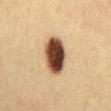Recorded during total-body skin imaging; not selected for excision or biopsy. The total-body-photography lesion software estimated an average lesion color of about L≈42 a*≈18 b*≈29 (CIELAB) and about 25 CIELAB-L* units darker than the surrounding skin. The software also gave a nevus-likeness score of about 100/100 and a lesion-detection confidence of about 100/100. The patient is a female aged approximately 30. The tile uses cross-polarized illumination. Cropped from a whole-body photographic skin survey; the tile spans about 15 mm. On the front of the torso.